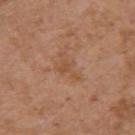Captured during whole-body skin photography for melanoma surveillance; the lesion was not biopsied.
A male patient in their mid-70s.
Longest diameter approximately 4 mm.
Automated image analysis of the tile measured a footprint of about 4.5 mm² and an eccentricity of roughly 0.85. It also reported an average lesion color of about L≈51 a*≈21 b*≈33 (CIELAB) and a lesion–skin lightness drop of about 6. The software also gave a border-irregularity index near 8/10, internal color variation of about 1 on a 0–10 scale, and peripheral color asymmetry of about 0.5. And it measured an automated nevus-likeness rating near 0 out of 100 and a detector confidence of about 100 out of 100 that the crop contains a lesion.
Located on the left upper arm.
A close-up tile cropped from a whole-body skin photograph, about 15 mm across.